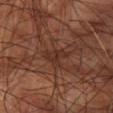Imaged during a routine full-body skin examination; the lesion was not biopsied and no histopathology is available. From the arm. A male subject, in their 60s. Cropped from a whole-body photographic skin survey; the tile spans about 15 mm.